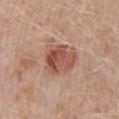Q: Was a biopsy performed?
A: total-body-photography surveillance lesion; no biopsy
Q: How was the tile lit?
A: white-light
Q: Lesion location?
A: the mid back
Q: Patient demographics?
A: male, aged 48 to 52
Q: How was this image acquired?
A: total-body-photography crop, ~15 mm field of view